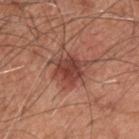Q: Was this lesion biopsied?
A: total-body-photography surveillance lesion; no biopsy
Q: Where on the body is the lesion?
A: the back
Q: What are the patient's age and sex?
A: male, aged around 60
Q: How large is the lesion?
A: about 3.5 mm
Q: What is the imaging modality?
A: total-body-photography crop, ~15 mm field of view
Q: How was the tile lit?
A: white-light illumination